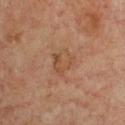Assessment:
Captured during whole-body skin photography for melanoma surveillance; the lesion was not biopsied.
Clinical summary:
Automated image analysis of the tile measured a border-irregularity index near 3/10 and a color-variation rating of about 4/10. Captured under cross-polarized illumination. The lesion is on the front of the torso. Longest diameter approximately 3.5 mm. A 15 mm crop from a total-body photograph taken for skin-cancer surveillance. A female subject in their mid- to late 40s.